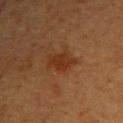biopsy status: total-body-photography surveillance lesion; no biopsy
subject: female, roughly 40 years of age
body site: the right upper arm
image: ~15 mm tile from a whole-body skin photo